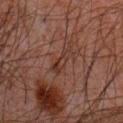Case summary:
* biopsy status: imaged on a skin check; not biopsied
* acquisition: 15 mm crop, total-body photography
* subject: male, roughly 60 years of age
* automated metrics: a lesion color around L≈26 a*≈15 b*≈20 in CIELAB, a lesion–skin lightness drop of about 5, and a lesion-to-skin contrast of about 6 (normalized; higher = more distinct); a border-irregularity index near 5/10, a within-lesion color-variation index near 2.5/10, and a peripheral color-asymmetry measure near 0.5
* site: the chest
* tile lighting: cross-polarized